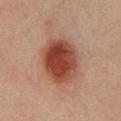| field | value |
|---|---|
| biopsy status | no biopsy performed (imaged during a skin exam) |
| illumination | cross-polarized illumination |
| image source | ~15 mm tile from a whole-body skin photo |
| subject | male, about 50 years old |
| diameter | ~6.5 mm (longest diameter) |
| automated metrics | a footprint of about 21 mm², an outline eccentricity of about 0.75 (0 = round, 1 = elongated), and two-axis asymmetry of about 0.1; a detector confidence of about 100 out of 100 that the crop contains a lesion |
| site | the chest |